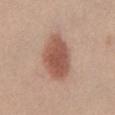lesion diameter = about 6 mm
illumination = white-light illumination
image source = 15 mm crop, total-body photography
patient = female, aged approximately 40
anatomic site = the chest